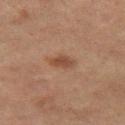Part of a total-body skin-imaging series; this lesion was reviewed on a skin check and was not flagged for biopsy. Approximately 3 mm at its widest. This image is a 15 mm lesion crop taken from a total-body photograph. The lesion is located on the leg. The tile uses cross-polarized illumination. The subject is a female aged 58–62.